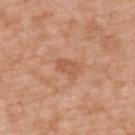{
  "biopsy_status": "not biopsied; imaged during a skin examination",
  "site": "upper back",
  "lesion_size": {
    "long_diameter_mm_approx": 3.0
  },
  "lighting": "white-light",
  "image": {
    "source": "total-body photography crop",
    "field_of_view_mm": 15
  },
  "automated_metrics": {
    "cielab_L": 56,
    "cielab_a": 24,
    "cielab_b": 35,
    "vs_skin_darker_L": 8.0,
    "vs_skin_contrast_norm": 5.5,
    "border_irregularity_0_10": 3.0,
    "color_variation_0_10": 3.0,
    "peripheral_color_asymmetry": 1.0
  },
  "patient": {
    "sex": "male",
    "age_approx": 50
  }
}